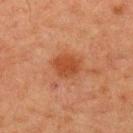Q: Was a biopsy performed?
A: no biopsy performed (imaged during a skin exam)
Q: Patient demographics?
A: male, in their 60s
Q: What is the lesion's diameter?
A: about 3.5 mm
Q: Lesion location?
A: the upper back
Q: How was this image acquired?
A: ~15 mm tile from a whole-body skin photo